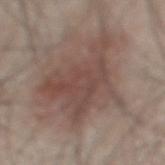Assessment: This lesion was catalogued during total-body skin photography and was not selected for biopsy. Background: A male patient in their mid- to late 40s. From the mid back. A 15 mm close-up extracted from a 3D total-body photography capture.